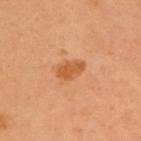This lesion was catalogued during total-body skin photography and was not selected for biopsy. The total-body-photography lesion software estimated a border-irregularity index near 2/10 and internal color variation of about 1.5 on a 0–10 scale. The software also gave a nevus-likeness score of about 55/100 and lesion-presence confidence of about 100/100. About 3 mm across. This is a cross-polarized tile. A close-up tile cropped from a whole-body skin photograph, about 15 mm across. The patient is a female aged approximately 60. From the chest.